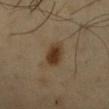Part of a total-body skin-imaging series; this lesion was reviewed on a skin check and was not flagged for biopsy. The tile uses cross-polarized illumination. A male patient about 50 years old. Measured at roughly 4 mm in maximum diameter. The lesion is on the chest. A 15 mm crop from a total-body photograph taken for skin-cancer surveillance.